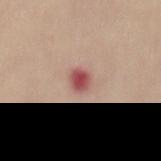<tbp_lesion>
<biopsy_status>not biopsied; imaged during a skin examination</biopsy_status>
<site>mid back</site>
<lesion_size>
  <long_diameter_mm_approx>2.5</long_diameter_mm_approx>
</lesion_size>
<lighting>white-light</lighting>
<patient>
  <sex>female</sex>
  <age_approx>55</age_approx>
</patient>
<image>
  <source>total-body photography crop</source>
  <field_of_view_mm>15</field_of_view_mm>
</image>
<automated_metrics>
  <vs_skin_darker_L>14.0</vs_skin_darker_L>
  <vs_skin_contrast_norm>9.5</vs_skin_contrast_norm>
</automated_metrics>
</tbp_lesion>